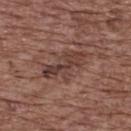Captured during whole-body skin photography for melanoma surveillance; the lesion was not biopsied.
Captured under white-light illumination.
The lesion is on the back.
The recorded lesion diameter is about 6 mm.
A 15 mm close-up tile from a total-body photography series done for melanoma screening.
The patient is a female roughly 75 years of age.
The lesion-visualizer software estimated a border-irregularity index near 6/10 and a within-lesion color-variation index near 5.5/10.